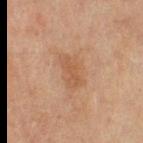Imaged during a routine full-body skin examination; the lesion was not biopsied and no histopathology is available. Imaged with cross-polarized lighting. Cropped from a whole-body photographic skin survey; the tile spans about 15 mm. Located on the right thigh. A female patient in their mid- to late 50s.